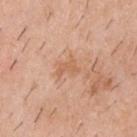Impression: This lesion was catalogued during total-body skin photography and was not selected for biopsy. Background: Longest diameter approximately 3 mm. A close-up tile cropped from a whole-body skin photograph, about 15 mm across. Imaged with white-light lighting. On the upper back. A male subject in their 40s. Automated tile analysis of the lesion measured a symmetry-axis asymmetry near 0.55. The analysis additionally found internal color variation of about 0 on a 0–10 scale and peripheral color asymmetry of about 0.